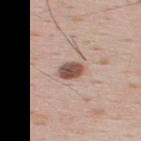notes: total-body-photography surveillance lesion; no biopsy | illumination: white-light illumination | automated lesion analysis: a footprint of about 4.5 mm², an eccentricity of roughly 0.65, and two-axis asymmetry of about 0.15; border irregularity of about 1 on a 0–10 scale, a within-lesion color-variation index near 4/10, and peripheral color asymmetry of about 1; an automated nevus-likeness rating near 95 out of 100 and a lesion-detection confidence of about 100/100 | subject: male, aged approximately 35 | lesion size: ≈2.5 mm | anatomic site: the upper back | acquisition: ~15 mm tile from a whole-body skin photo.